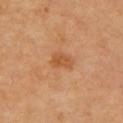Captured during whole-body skin photography for melanoma surveillance; the lesion was not biopsied.
Captured under cross-polarized illumination.
Longest diameter approximately 3 mm.
The lesion is on the left upper arm.
The patient is a female aged approximately 60.
A 15 mm close-up extracted from a 3D total-body photography capture.